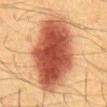notes: catalogued during a skin exam; not biopsied | subject: male, roughly 60 years of age | tile lighting: cross-polarized illumination | image source: ~15 mm tile from a whole-body skin photo | size: ~11 mm (longest diameter) | anatomic site: the abdomen.